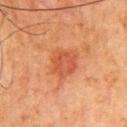workup = imaged on a skin check; not biopsied | image source = total-body-photography crop, ~15 mm field of view | subject = male, aged approximately 80 | lighting = cross-polarized | location = the chest.